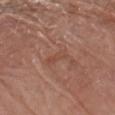workup = catalogued during a skin exam; not biopsied
image = ~15 mm tile from a whole-body skin photo
diameter = ~2.5 mm (longest diameter)
image-analysis metrics = an automated nevus-likeness rating near 0 out of 100 and a detector confidence of about 100 out of 100 that the crop contains a lesion
tile lighting = white-light
body site = the left forearm
subject = male, aged 78–82